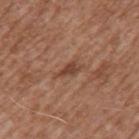| feature | finding |
|---|---|
| notes | imaged on a skin check; not biopsied |
| lesion size | about 2.5 mm |
| patient | male, roughly 75 years of age |
| imaging modality | ~15 mm crop, total-body skin-cancer survey |
| image-analysis metrics | a lesion area of about 3 mm² and an eccentricity of roughly 0.9; internal color variation of about 1 on a 0–10 scale and radial color variation of about 0 |
| body site | the mid back |
| illumination | white-light illumination |